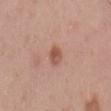Q: Was a biopsy performed?
A: total-body-photography surveillance lesion; no biopsy
Q: How was this image acquired?
A: ~15 mm crop, total-body skin-cancer survey
Q: Where on the body is the lesion?
A: the mid back
Q: How was the tile lit?
A: white-light illumination
Q: What are the patient's age and sex?
A: male, aged approximately 50
Q: How large is the lesion?
A: ~2.5 mm (longest diameter)